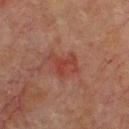biopsy_status: not biopsied; imaged during a skin examination
lighting: cross-polarized
lesion_size:
  long_diameter_mm_approx: 3.5
automated_metrics:
  area_mm2_approx: 7.0
  shape_asymmetry: 0.4
  nevus_likeness_0_100: 0
  lesion_detection_confidence_0_100: 100
site: upper back
patient:
  sex: male
  age_approx: 65
image:
  source: total-body photography crop
  field_of_view_mm: 15